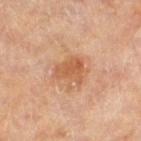{
  "biopsy_status": "not biopsied; imaged during a skin examination"
}